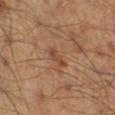The patient is a male about 45 years old. On the leg. Cropped from a whole-body photographic skin survey; the tile spans about 15 mm. An algorithmic analysis of the crop reported a border-irregularity index near 3.5/10, a color-variation rating of about 0/10, and peripheral color asymmetry of about 0. It also reported a detector confidence of about 100 out of 100 that the crop contains a lesion. Approximately 3 mm at its widest.